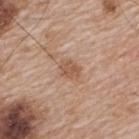| key | value |
|---|---|
| workup | no biopsy performed (imaged during a skin exam) |
| lighting | white-light |
| image | ~15 mm crop, total-body skin-cancer survey |
| patient | male, aged 63–67 |
| location | the upper back |
| lesion size | ~2.5 mm (longest diameter) |
| image-analysis metrics | a footprint of about 4 mm², a shape eccentricity near 0.7, and a symmetry-axis asymmetry near 0.25; an average lesion color of about L≈55 a*≈20 b*≈31 (CIELAB) and a lesion-to-skin contrast of about 6.5 (normalized; higher = more distinct); lesion-presence confidence of about 100/100 |